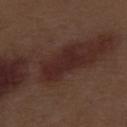workup: catalogued during a skin exam; not biopsied
lighting: white-light
image source: 15 mm crop, total-body photography
location: the right thigh
patient: male, aged approximately 70